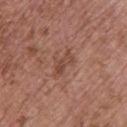Findings:
* biopsy status · total-body-photography surveillance lesion; no biopsy
* patient · female, about 65 years old
* illumination · white-light
* acquisition · ~15 mm tile from a whole-body skin photo
* image-analysis metrics · an area of roughly 5 mm² and an eccentricity of roughly 0.85; a mean CIELAB color near L≈46 a*≈22 b*≈27 and a normalized border contrast of about 6; a border-irregularity rating of about 5/10, a within-lesion color-variation index near 3.5/10, and a peripheral color-asymmetry measure near 1.5; an automated nevus-likeness rating near 0 out of 100 and a detector confidence of about 100 out of 100 that the crop contains a lesion
* size · about 3.5 mm
* body site · the upper back